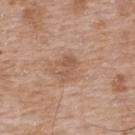workup: catalogued during a skin exam; not biopsied | imaging modality: ~15 mm tile from a whole-body skin photo | location: the upper back | size: ≈3 mm | lighting: white-light | subject: male, aged around 50.